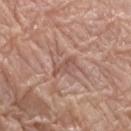Impression: No biopsy was performed on this lesion — it was imaged during a full skin examination and was not determined to be concerning. Context: Automated image analysis of the tile measured a lesion area of about 2.5 mm². And it measured a border-irregularity index near 5.5/10, internal color variation of about 0 on a 0–10 scale, and peripheral color asymmetry of about 0. It also reported an automated nevus-likeness rating near 0 out of 100 and a lesion-detection confidence of about 65/100. The recorded lesion diameter is about 3 mm. A female patient roughly 80 years of age. The tile uses white-light illumination. On the right forearm. Cropped from a total-body skin-imaging series; the visible field is about 15 mm.